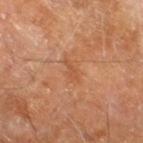| field | value |
|---|---|
| workup | catalogued during a skin exam; not biopsied |
| lesion diameter | ≈3 mm |
| lighting | cross-polarized illumination |
| subject | male, roughly 60 years of age |
| body site | the leg |
| acquisition | ~15 mm tile from a whole-body skin photo |